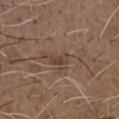Located on the chest. A male subject aged around 50. A roughly 15 mm field-of-view crop from a total-body skin photograph.